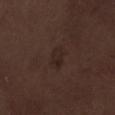image source = ~15 mm tile from a whole-body skin photo | tile lighting = white-light | automated lesion analysis = a lesion area of about 4 mm² and a shape eccentricity near 0.75; a border-irregularity index near 2.5/10, a color-variation rating of about 2.5/10, and radial color variation of about 1 | subject = male, aged approximately 70 | site = the leg.